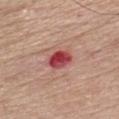Q: Automated lesion metrics?
A: an eccentricity of roughly 0.7; a border-irregularity index near 1.5/10 and internal color variation of about 8 on a 0–10 scale
Q: What is the imaging modality?
A: ~15 mm tile from a whole-body skin photo
Q: What are the patient's age and sex?
A: male, in their mid- to late 50s
Q: Lesion location?
A: the front of the torso
Q: Illumination type?
A: white-light
Q: What is the lesion's diameter?
A: about 3.5 mm